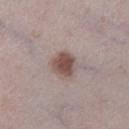Findings:
* tile lighting · white-light
* imaging modality · total-body-photography crop, ~15 mm field of view
* subject · male, aged approximately 40
* diameter · ≈3 mm
* TBP lesion metrics · an area of roughly 7 mm² and an outline eccentricity of about 0.2 (0 = round, 1 = elongated)
* body site · the right lower leg